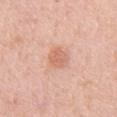Background:
A region of skin cropped from a whole-body photographic capture, roughly 15 mm wide. The patient is a male aged around 55. The lesion is located on the abdomen. This is a white-light tile.